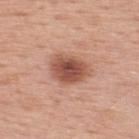Notes:
– image-analysis metrics — a lesion color around L≈52 a*≈25 b*≈29 in CIELAB and roughly 14 lightness units darker than nearby skin; a border-irregularity index near 1.5/10, internal color variation of about 5 on a 0–10 scale, and radial color variation of about 1; a lesion-detection confidence of about 100/100
– acquisition — ~15 mm crop, total-body skin-cancer survey
– lighting — white-light
– site — the upper back
– subject — male, approximately 65 years of age